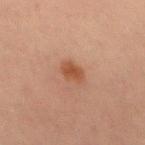Impression: The lesion was tiled from a total-body skin photograph and was not biopsied. Acquisition and patient details: A male patient about 30 years old. A region of skin cropped from a whole-body photographic capture, roughly 15 mm wide. Located on the back. The lesion's longest dimension is about 2.5 mm. Captured under cross-polarized illumination.